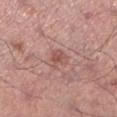Image and clinical context: A lesion tile, about 15 mm wide, cut from a 3D total-body photograph. Longest diameter approximately 2.5 mm. Captured under white-light illumination. The lesion is located on the leg. The subject is a male in their 40s.